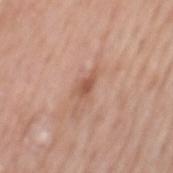Recorded during total-body skin imaging; not selected for excision or biopsy. A region of skin cropped from a whole-body photographic capture, roughly 15 mm wide. The lesion is on the mid back. A female patient, about 60 years old. Captured under white-light illumination. The lesion's longest dimension is about 3 mm.